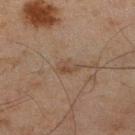Q: Was this lesion biopsied?
A: total-body-photography surveillance lesion; no biopsy
Q: Who is the patient?
A: male, aged around 45
Q: How large is the lesion?
A: about 3.5 mm
Q: What kind of image is this?
A: ~15 mm crop, total-body skin-cancer survey
Q: What is the anatomic site?
A: the right lower leg